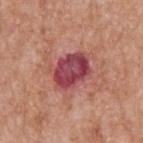follow-up: imaged on a skin check; not biopsied | image: ~15 mm crop, total-body skin-cancer survey | automated lesion analysis: an eccentricity of roughly 0.6; an average lesion color of about L≈45 a*≈33 b*≈20 (CIELAB) and a normalized lesion–skin contrast near 12; border irregularity of about 2 on a 0–10 scale and a color-variation rating of about 7/10; a classifier nevus-likeness of about 0/100 and a detector confidence of about 100 out of 100 that the crop contains a lesion | illumination: white-light illumination | patient: male, aged approximately 60 | anatomic site: the upper back.